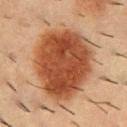Findings:
• biopsy status · no biopsy performed (imaged during a skin exam)
• subject · male, roughly 55 years of age
• automated lesion analysis · an average lesion color of about L≈42 a*≈24 b*≈32 (CIELAB), a lesion–skin lightness drop of about 15, and a normalized lesion–skin contrast near 11.5; internal color variation of about 5 on a 0–10 scale and a peripheral color-asymmetry measure near 1.5; a classifier nevus-likeness of about 100/100 and lesion-presence confidence of about 100/100
• imaging modality · ~15 mm crop, total-body skin-cancer survey
• size · ~8.5 mm (longest diameter)
• site · the upper back
• illumination · cross-polarized illumination